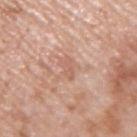The lesion was photographed on a routine skin check and not biopsied; there is no pathology result. The patient is a male aged 68–72. The lesion-visualizer software estimated a lesion–skin lightness drop of about 7 and a normalized border contrast of about 4.5. The software also gave a classifier nevus-likeness of about 0/100 and lesion-presence confidence of about 100/100. The tile uses white-light illumination. A region of skin cropped from a whole-body photographic capture, roughly 15 mm wide. On the right upper arm.